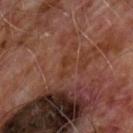notes: no biopsy performed (imaged during a skin exam)
illumination: cross-polarized illumination
subject: male, in their 60s
imaging modality: total-body-photography crop, ~15 mm field of view
TBP lesion metrics: a footprint of about 3 mm², a shape eccentricity near 0.9, and a shape-asymmetry score of about 0.35 (0 = symmetric); a nevus-likeness score of about 0/100 and lesion-presence confidence of about 70/100
site: the chest
diameter: ~3 mm (longest diameter)